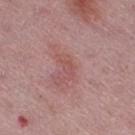<case>
<biopsy_status>not biopsied; imaged during a skin examination</biopsy_status>
<site>right thigh</site>
<image>
  <source>total-body photography crop</source>
  <field_of_view_mm>15</field_of_view_mm>
</image>
<patient>
  <sex>female</sex>
  <age_approx>45</age_approx>
</patient>
</case>